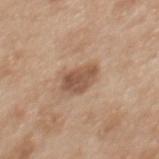Imaged during a routine full-body skin examination; the lesion was not biopsied and no histopathology is available.
Longest diameter approximately 3.5 mm.
From the back.
The patient is a female in their 40s.
Imaged with white-light lighting.
Cropped from a total-body skin-imaging series; the visible field is about 15 mm.
The total-body-photography lesion software estimated an eccentricity of roughly 0.7 and a shape-asymmetry score of about 0.2 (0 = symmetric). It also reported a mean CIELAB color near L≈53 a*≈19 b*≈30, a lesion–skin lightness drop of about 11, and a normalized lesion–skin contrast near 8. The analysis additionally found a classifier nevus-likeness of about 20/100 and a lesion-detection confidence of about 100/100.